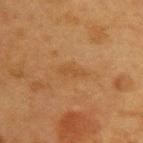patient:
  sex: female
  age_approx: 40
lesion_size:
  long_diameter_mm_approx: 3.0
image:
  source: total-body photography crop
  field_of_view_mm: 15
site: upper back
lighting: cross-polarized